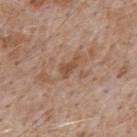Part of a total-body skin-imaging series; this lesion was reviewed on a skin check and was not flagged for biopsy.
A male patient, about 50 years old.
An algorithmic analysis of the crop reported an average lesion color of about L≈50 a*≈20 b*≈30 (CIELAB), a lesion–skin lightness drop of about 8, and a normalized border contrast of about 6.5. And it measured a border-irregularity index near 5/10, a within-lesion color-variation index near 0.5/10, and radial color variation of about 0.
Measured at roughly 2.5 mm in maximum diameter.
Located on the upper back.
A 15 mm close-up extracted from a 3D total-body photography capture.